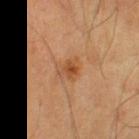Clinical impression: Part of a total-body skin-imaging series; this lesion was reviewed on a skin check and was not flagged for biopsy. Image and clinical context: Captured under cross-polarized illumination. The lesion-visualizer software estimated a lesion area of about 4.5 mm², a shape eccentricity near 0.6, and a shape-asymmetry score of about 0.45 (0 = symmetric). The software also gave a lesion-to-skin contrast of about 6.5 (normalized; higher = more distinct). Located on the left thigh. Cropped from a total-body skin-imaging series; the visible field is about 15 mm. A male subject, aged approximately 70. Approximately 3 mm at its widest.